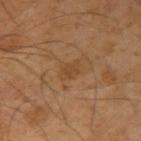  biopsy_status: not biopsied; imaged during a skin examination
  lesion_size:
    long_diameter_mm_approx: 2.5
  image:
    source: total-body photography crop
    field_of_view_mm: 15
  automated_metrics:
    eccentricity: 0.8
    shape_asymmetry: 0.25
    color_variation_0_10: 1.5
    peripheral_color_asymmetry: 0.5
  lighting: cross-polarized
  patient:
    sex: male
    age_approx: 50
  site: left arm